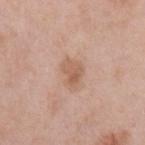Acquisition and patient details:
The lesion-visualizer software estimated a lesion color around L≈59 a*≈20 b*≈31 in CIELAB, roughly 9 lightness units darker than nearby skin, and a normalized border contrast of about 6.5. The software also gave a classifier nevus-likeness of about 5/100 and a lesion-detection confidence of about 100/100. Cropped from a whole-body photographic skin survey; the tile spans about 15 mm. The patient is a female in their 40s. Located on the left upper arm. Approximately 3.5 mm at its widest.